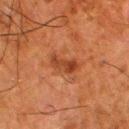Q: Is there a histopathology result?
A: catalogued during a skin exam; not biopsied
Q: Where on the body is the lesion?
A: the leg
Q: What kind of image is this?
A: ~15 mm crop, total-body skin-cancer survey
Q: Illumination type?
A: cross-polarized
Q: Patient demographics?
A: male, approximately 80 years of age